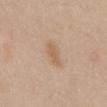follow-up = imaged on a skin check; not biopsied
body site = the abdomen
acquisition = ~15 mm tile from a whole-body skin photo
patient = male, approximately 30 years of age